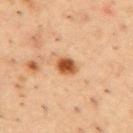- biopsy status — total-body-photography surveillance lesion; no biopsy
- tile lighting — cross-polarized
- lesion diameter — ~2.5 mm (longest diameter)
- patient — male, approximately 55 years of age
- TBP lesion metrics — a footprint of about 4.5 mm²; a mean CIELAB color near L≈54 a*≈26 b*≈40 and roughly 16 lightness units darker than nearby skin; a border-irregularity index near 1.5/10, internal color variation of about 5.5 on a 0–10 scale, and peripheral color asymmetry of about 1.5
- image source — total-body-photography crop, ~15 mm field of view
- body site — the mid back